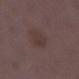No biopsy was performed on this lesion — it was imaged during a full skin examination and was not determined to be concerning.
The lesion is on the right lower leg.
Approximately 3 mm at its widest.
Imaged with white-light lighting.
An algorithmic analysis of the crop reported a footprint of about 5.5 mm² and an outline eccentricity of about 0.65 (0 = round, 1 = elongated). And it measured a nevus-likeness score of about 10/100.
A female patient aged approximately 35.
A 15 mm close-up tile from a total-body photography series done for melanoma screening.